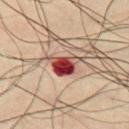The lesion was photographed on a routine skin check and not biopsied; there is no pathology result. An algorithmic analysis of the crop reported an outline eccentricity of about 0.75 (0 = round, 1 = elongated) and a symmetry-axis asymmetry near 0.45. The software also gave about 21 CIELAB-L* units darker than the surrounding skin. The analysis additionally found border irregularity of about 4.5 on a 0–10 scale and radial color variation of about 3.5. On the chest. A male subject in their 50s. This is a cross-polarized tile. A region of skin cropped from a whole-body photographic capture, roughly 15 mm wide. The recorded lesion diameter is about 4.5 mm.